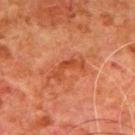Assessment: This lesion was catalogued during total-body skin photography and was not selected for biopsy. Image and clinical context: The subject is a male aged around 80. From the upper back. Cropped from a whole-body photographic skin survey; the tile spans about 15 mm.